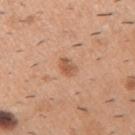<case>
  <biopsy_status>not biopsied; imaged during a skin examination</biopsy_status>
  <patient>
    <sex>male</sex>
    <age_approx>40</age_approx>
  </patient>
  <lesion_size>
    <long_diameter_mm_approx>2.5</long_diameter_mm_approx>
  </lesion_size>
  <site>arm</site>
  <image>
    <source>total-body photography crop</source>
    <field_of_view_mm>15</field_of_view_mm>
  </image>
</case>